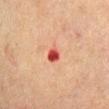workup — catalogued during a skin exam; not biopsied
patient — male, approximately 70 years of age
acquisition — 15 mm crop, total-body photography
anatomic site — the right upper arm
lesion size — ~3 mm (longest diameter)
illumination — cross-polarized illumination
image-analysis metrics — border irregularity of about 2 on a 0–10 scale and internal color variation of about 10 on a 0–10 scale; a nevus-likeness score of about 0/100